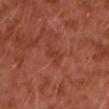Imaged during a routine full-body skin examination; the lesion was not biopsied and no histopathology is available. A male patient, approximately 30 years of age. This is a cross-polarized tile. A close-up tile cropped from a whole-body skin photograph, about 15 mm across. About 2.5 mm across. The lesion is on the left upper arm.